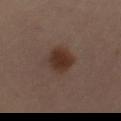| key | value |
|---|---|
| follow-up | imaged on a skin check; not biopsied |
| image-analysis metrics | a lesion area of about 10 mm² and a symmetry-axis asymmetry near 0.15; border irregularity of about 2 on a 0–10 scale, a color-variation rating of about 3/10, and peripheral color asymmetry of about 1; a lesion-detection confidence of about 100/100 |
| body site | the left thigh |
| acquisition | 15 mm crop, total-body photography |
| patient | female, approximately 40 years of age |